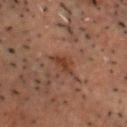The lesion was photographed on a routine skin check and not biopsied; there is no pathology result. The lesion is on the head or neck. A male patient aged 48 to 52. A lesion tile, about 15 mm wide, cut from a 3D total-body photograph.